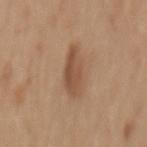Clinical impression: Imaged during a routine full-body skin examination; the lesion was not biopsied and no histopathology is available. Acquisition and patient details: About 5 mm across. This image is a 15 mm lesion crop taken from a total-body photograph. A female subject in their 40s. The lesion is located on the mid back.